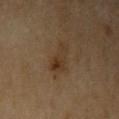Q: Was a biopsy performed?
A: no biopsy performed (imaged during a skin exam)
Q: Who is the patient?
A: male, aged around 70
Q: Illumination type?
A: cross-polarized illumination
Q: What did automated image analysis measure?
A: a lesion area of about 6.5 mm² and a symmetry-axis asymmetry near 0.4; a lesion color around L≈32 a*≈14 b*≈27 in CIELAB, about 6 CIELAB-L* units darker than the surrounding skin, and a normalized border contrast of about 7
Q: How large is the lesion?
A: about 4.5 mm
Q: Lesion location?
A: the left upper arm
Q: What is the imaging modality?
A: 15 mm crop, total-body photography